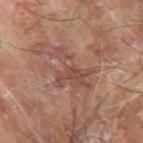Part of a total-body skin-imaging series; this lesion was reviewed on a skin check and was not flagged for biopsy. A male patient, aged approximately 65. Located on the arm. Cropped from a whole-body photographic skin survey; the tile spans about 15 mm.